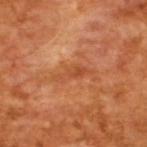biopsy status = imaged on a skin check; not biopsied
lighting = cross-polarized illumination
patient = male, aged approximately 65
image = ~15 mm tile from a whole-body skin photo